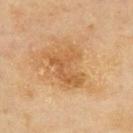This lesion was catalogued during total-body skin photography and was not selected for biopsy. On the upper back. The subject is a female approximately 60 years of age. Imaged with cross-polarized lighting. Cropped from a whole-body photographic skin survey; the tile spans about 15 mm.